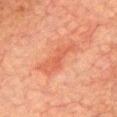Assessment:
The lesion was tiled from a total-body skin photograph and was not biopsied.
Background:
The lesion is located on the front of the torso. A male subject approximately 75 years of age. A 15 mm close-up extracted from a 3D total-body photography capture.